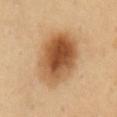notes = catalogued during a skin exam; not biopsied
subject = female, about 50 years old
body site = the abdomen
imaging modality = ~15 mm tile from a whole-body skin photo
illumination = cross-polarized
lesion size = ~7 mm (longest diameter)